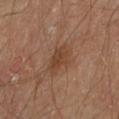Recorded during total-body skin imaging; not selected for excision or biopsy. Longest diameter approximately 3.5 mm. A 15 mm close-up tile from a total-body photography series done for melanoma screening. The subject is a male in their mid- to late 60s. The tile uses cross-polarized illumination. From the left upper arm.